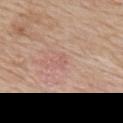Case summary:
- body site — the mid back
- tile lighting — white-light illumination
- subject — male, aged around 60
- imaging modality — total-body-photography crop, ~15 mm field of view
- pathology — a superficial basal cell carcinoma